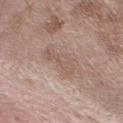Recorded during total-body skin imaging; not selected for excision or biopsy.
From the arm.
A male patient roughly 70 years of age.
A region of skin cropped from a whole-body photographic capture, roughly 15 mm wide.